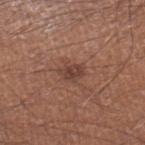{"biopsy_status": "not biopsied; imaged during a skin examination", "lesion_size": {"long_diameter_mm_approx": 2.5}, "patient": {"sex": "male", "age_approx": 45}, "site": "leg", "image": {"source": "total-body photography crop", "field_of_view_mm": 15}, "lighting": "white-light", "automated_metrics": {"area_mm2_approx": 4.0, "eccentricity": 0.7, "cielab_L": 41, "cielab_a": 21, "cielab_b": 24, "vs_skin_darker_L": 9.0, "vs_skin_contrast_norm": 7.5}}